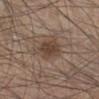Part of a total-body skin-imaging series; this lesion was reviewed on a skin check and was not flagged for biopsy. Located on the leg. A male patient, in their mid- to late 40s. A roughly 15 mm field-of-view crop from a total-body skin photograph.